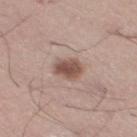Q: Is there a histopathology result?
A: catalogued during a skin exam; not biopsied
Q: Lesion location?
A: the left lower leg
Q: Who is the patient?
A: male, aged around 35
Q: Automated lesion metrics?
A: a classifier nevus-likeness of about 95/100 and a detector confidence of about 100 out of 100 that the crop contains a lesion
Q: How was this image acquired?
A: ~15 mm crop, total-body skin-cancer survey
Q: What lighting was used for the tile?
A: white-light illumination